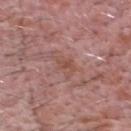follow-up = imaged on a skin check; not biopsied
subject = male, about 60 years old
body site = the head or neck
imaging modality = total-body-photography crop, ~15 mm field of view